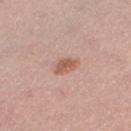<record>
<biopsy_status>not biopsied; imaged during a skin examination</biopsy_status>
<patient>
  <sex>female</sex>
  <age_approx>35</age_approx>
</patient>
<image>
  <source>total-body photography crop</source>
  <field_of_view_mm>15</field_of_view_mm>
</image>
<site>left thigh</site>
</record>